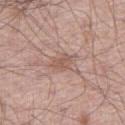Q: Was this lesion biopsied?
A: no biopsy performed (imaged during a skin exam)
Q: What is the imaging modality?
A: total-body-photography crop, ~15 mm field of view
Q: Patient demographics?
A: male, aged 58–62
Q: What did automated image analysis measure?
A: a lesion area of about 4 mm² and a shape-asymmetry score of about 0.3 (0 = symmetric); an average lesion color of about L≈55 a*≈19 b*≈26 (CIELAB), roughly 8 lightness units darker than nearby skin, and a normalized lesion–skin contrast near 6; a lesion-detection confidence of about 100/100
Q: What lighting was used for the tile?
A: white-light
Q: What is the lesion's diameter?
A: ~2.5 mm (longest diameter)
Q: Lesion location?
A: the right thigh